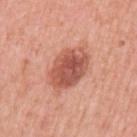Located on the left upper arm. This is a white-light tile. The patient is a female about 40 years old. This image is a 15 mm lesion crop taken from a total-body photograph.